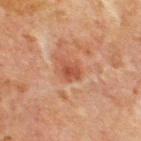{"biopsy_status": "not biopsied; imaged during a skin examination", "automated_metrics": {"area_mm2_approx": 4.5, "eccentricity": 0.65, "shape_asymmetry": 0.2, "cielab_L": 41, "cielab_a": 23, "cielab_b": 28, "vs_skin_darker_L": 8.0, "border_irregularity_0_10": 2.0, "color_variation_0_10": 2.5, "peripheral_color_asymmetry": 1.0}, "lighting": "cross-polarized", "lesion_size": {"long_diameter_mm_approx": 3.0}, "image": {"source": "total-body photography crop", "field_of_view_mm": 15}, "patient": {"sex": "male", "age_approx": 65}, "site": "chest"}